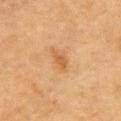Clinical impression: Recorded during total-body skin imaging; not selected for excision or biopsy. Acquisition and patient details: The total-body-photography lesion software estimated an average lesion color of about L≈51 a*≈20 b*≈39 (CIELAB), about 8 CIELAB-L* units darker than the surrounding skin, and a normalized lesion–skin contrast near 6.5. It also reported a border-irregularity index near 3.5/10, a color-variation rating of about 1.5/10, and radial color variation of about 0.5. The subject is a male about 85 years old or older. This is a cross-polarized tile. A close-up tile cropped from a whole-body skin photograph, about 15 mm across. The lesion is on the chest. Measured at roughly 3 mm in maximum diameter.